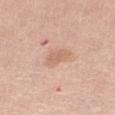Q: Was a biopsy performed?
A: total-body-photography surveillance lesion; no biopsy
Q: What lighting was used for the tile?
A: white-light illumination
Q: How was this image acquired?
A: ~15 mm crop, total-body skin-cancer survey
Q: What are the patient's age and sex?
A: female, aged 63–67
Q: Lesion size?
A: ≈3 mm
Q: Lesion location?
A: the right thigh